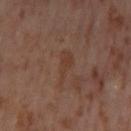This lesion was catalogued during total-body skin photography and was not selected for biopsy. Captured under cross-polarized illumination. The recorded lesion diameter is about 5 mm. A region of skin cropped from a whole-body photographic capture, roughly 15 mm wide. The patient is a female roughly 55 years of age. Located on the left thigh.